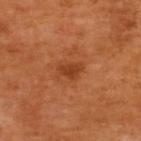The total-body-photography lesion software estimated a lesion area of about 3.5 mm², an outline eccentricity of about 0.75 (0 = round, 1 = elongated), and a symmetry-axis asymmetry near 0.35. And it measured a lesion color around L≈42 a*≈30 b*≈41 in CIELAB, a lesion–skin lightness drop of about 9, and a lesion-to-skin contrast of about 7 (normalized; higher = more distinct). And it measured a nevus-likeness score of about 35/100 and lesion-presence confidence of about 100/100.
A female subject, in their mid-50s.
The tile uses cross-polarized illumination.
A 15 mm crop from a total-body photograph taken for skin-cancer surveillance.
The recorded lesion diameter is about 2.5 mm.
The lesion is on the upper back.